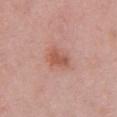Background: The lesion is on the chest. A 15 mm close-up tile from a total-body photography series done for melanoma screening. The patient is a female aged 33 to 37. The tile uses white-light illumination.